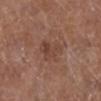Assessment: The lesion was photographed on a routine skin check and not biopsied; there is no pathology result. Acquisition and patient details: A female subject, aged around 80. The lesion is on the leg. A 15 mm crop from a total-body photograph taken for skin-cancer surveillance. The tile uses white-light illumination. Automated tile analysis of the lesion measured an average lesion color of about L≈42 a*≈20 b*≈26 (CIELAB) and roughly 7 lightness units darker than nearby skin. It also reported a border-irregularity index near 4.5/10, internal color variation of about 4 on a 0–10 scale, and a peripheral color-asymmetry measure near 1.5. The analysis additionally found a classifier nevus-likeness of about 0/100 and lesion-presence confidence of about 100/100. Longest diameter approximately 3.5 mm.